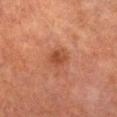No biopsy was performed on this lesion — it was imaged during a full skin examination and was not determined to be concerning.
On the left lower leg.
The total-body-photography lesion software estimated a lesion area of about 4.5 mm², a shape eccentricity near 0.75, and two-axis asymmetry of about 0.25. It also reported a border-irregularity index near 2.5/10, a within-lesion color-variation index near 2.5/10, and a peripheral color-asymmetry measure near 1.
Cropped from a total-body skin-imaging series; the visible field is about 15 mm.
A male subject, aged approximately 70.
The lesion's longest dimension is about 3 mm.